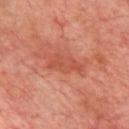Recorded during total-body skin imaging; not selected for excision or biopsy.
Automated tile analysis of the lesion measured a footprint of about 5.5 mm², an eccentricity of roughly 0.9, and a shape-asymmetry score of about 0.5 (0 = symmetric). And it measured a mean CIELAB color near L≈50 a*≈31 b*≈33. The analysis additionally found a border-irregularity rating of about 7/10, a color-variation rating of about 0.5/10, and radial color variation of about 0.
A male patient, roughly 65 years of age.
This is a cross-polarized tile.
A 15 mm close-up extracted from a 3D total-body photography capture.
The lesion is on the chest.
The lesion's longest dimension is about 4 mm.